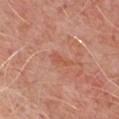No biopsy was performed on this lesion — it was imaged during a full skin examination and was not determined to be concerning. A male subject, aged approximately 50. On the front of the torso. A 15 mm close-up extracted from a 3D total-body photography capture.